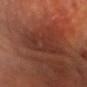A lesion tile, about 15 mm wide, cut from a 3D total-body photograph.
A male subject, in their mid-60s.
The lesion is on the head or neck.